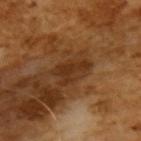workup: imaged on a skin check; not biopsied | subject: male, aged approximately 65 | tile lighting: cross-polarized | automated lesion analysis: a footprint of about 6.5 mm², an eccentricity of roughly 0.8, and two-axis asymmetry of about 0.55; an average lesion color of about L≈31 a*≈21 b*≈32 (CIELAB), about 8 CIELAB-L* units darker than the surrounding skin, and a normalized lesion–skin contrast near 8; a within-lesion color-variation index near 1/10 and peripheral color asymmetry of about 0.5; a classifier nevus-likeness of about 0/100 and a lesion-detection confidence of about 100/100 | acquisition: 15 mm crop, total-body photography.